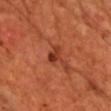Captured during whole-body skin photography for melanoma surveillance; the lesion was not biopsied. The patient is a male aged 63 to 67. The tile uses cross-polarized illumination. Approximately 2.5 mm at its widest. Cropped from a total-body skin-imaging series; the visible field is about 15 mm. Located on the front of the torso. Automated image analysis of the tile measured a lesion area of about 3.5 mm², a shape eccentricity near 0.75, and a symmetry-axis asymmetry near 0.3. It also reported lesion-presence confidence of about 100/100.